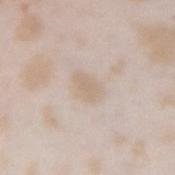acquisition: ~15 mm tile from a whole-body skin photo
patient: female, aged 23–27
site: the right forearm